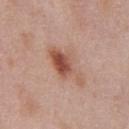- notes — no biopsy performed (imaged during a skin exam)
- patient — male, aged 53–57
- automated lesion analysis — lesion-presence confidence of about 100/100
- anatomic site — the chest
- imaging modality — 15 mm crop, total-body photography
- size — ~5.5 mm (longest diameter)